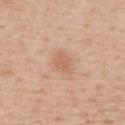Clinical impression:
No biopsy was performed on this lesion — it was imaged during a full skin examination and was not determined to be concerning.
Image and clinical context:
The subject is a male aged around 60. Located on the upper back. Imaged with white-light lighting. A 15 mm crop from a total-body photograph taken for skin-cancer surveillance.